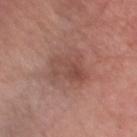Q: Was this lesion biopsied?
A: imaged on a skin check; not biopsied